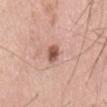The lesion was photographed on a routine skin check and not biopsied; there is no pathology result.
A male subject about 50 years old.
From the back.
Approximately 2.5 mm at its widest.
A region of skin cropped from a whole-body photographic capture, roughly 15 mm wide.
Captured under white-light illumination.